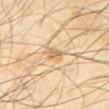About 2.5 mm across. Imaged with cross-polarized lighting. The patient is a male aged around 65. The lesion-visualizer software estimated a lesion area of about 4 mm², an eccentricity of roughly 0.65, and a symmetry-axis asymmetry near 0.3. The analysis additionally found an average lesion color of about L≈54 a*≈13 b*≈32 (CIELAB) and a lesion–skin lightness drop of about 8. It also reported a nevus-likeness score of about 5/100 and a detector confidence of about 95 out of 100 that the crop contains a lesion. Located on the abdomen. Cropped from a total-body skin-imaging series; the visible field is about 15 mm.